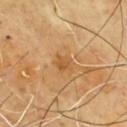<lesion>
  <biopsy_status>not biopsied; imaged during a skin examination</biopsy_status>
  <patient>
    <sex>male</sex>
    <age_approx>65</age_approx>
  </patient>
  <image>
    <source>total-body photography crop</source>
    <field_of_view_mm>15</field_of_view_mm>
  </image>
  <site>chest</site>
</lesion>